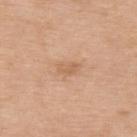Q: Was this lesion biopsied?
A: catalogued during a skin exam; not biopsied
Q: How was this image acquired?
A: ~15 mm crop, total-body skin-cancer survey
Q: Illumination type?
A: white-light illumination
Q: What are the patient's age and sex?
A: female, aged 73–77
Q: How large is the lesion?
A: ≈3 mm
Q: Lesion location?
A: the upper back
Q: Automated lesion metrics?
A: a lesion area of about 4 mm², an outline eccentricity of about 0.8 (0 = round, 1 = elongated), and a shape-asymmetry score of about 0.3 (0 = symmetric); a border-irregularity rating of about 2.5/10 and radial color variation of about 0.5